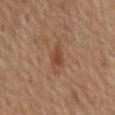| field | value |
|---|---|
| follow-up | catalogued during a skin exam; not biopsied |
| automated metrics | a footprint of about 3.5 mm², an outline eccentricity of about 0.85 (0 = round, 1 = elongated), and a shape-asymmetry score of about 0.3 (0 = symmetric); a lesion color around L≈41 a*≈21 b*≈29 in CIELAB and a normalized lesion–skin contrast near 7 |
| image source | ~15 mm tile from a whole-body skin photo |
| illumination | cross-polarized |
| size | ≈3 mm |
| site | the mid back |
| patient | male, aged 63–67 |